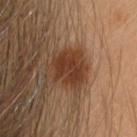Background: The subject is a female in their mid-20s. Captured under cross-polarized illumination. Cropped from a total-body skin-imaging series; the visible field is about 15 mm. The lesion is on the head or neck. Measured at roughly 4 mm in maximum diameter. Conclusion: Histopathology of the biopsied lesion showed an atypical melanocytic neoplasm, classified as a lesion of indeterminate malignant potential.